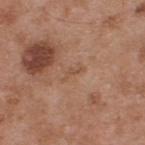follow-up = imaged on a skin check; not biopsied
subject = male, approximately 55 years of age
acquisition = total-body-photography crop, ~15 mm field of view
lighting = white-light
anatomic site = the upper back